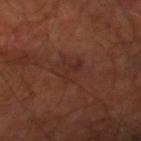biopsy_status: not biopsied; imaged during a skin examination
patient:
  age_approx: 65
site: right thigh
image:
  source: total-body photography crop
  field_of_view_mm: 15
lighting: cross-polarized